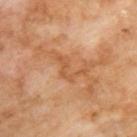Q: What is the anatomic site?
A: the upper back
Q: What is the imaging modality?
A: total-body-photography crop, ~15 mm field of view
Q: Illumination type?
A: cross-polarized
Q: Who is the patient?
A: male, approximately 70 years of age
Q: Lesion size?
A: about 4 mm